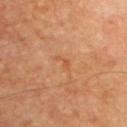biopsy_status: not biopsied; imaged during a skin examination
image:
  source: total-body photography crop
  field_of_view_mm: 15
site: upper back
lesion_size:
  long_diameter_mm_approx: 3.0
lighting: cross-polarized
automated_metrics:
  area_mm2_approx: 2.5
  eccentricity: 0.9
  shape_asymmetry: 0.55
  cielab_L: 55
  cielab_a: 24
  cielab_b: 39
  vs_skin_darker_L: 5.0
  vs_skin_contrast_norm: 4.5
  color_variation_0_10: 0.0
  nevus_likeness_0_100: 0
  lesion_detection_confidence_0_100: 100
patient:
  sex: male
  age_approx: 60